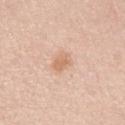<record>
  <site>chest</site>
  <lighting>white-light</lighting>
  <patient>
    <sex>female</sex>
    <age_approx>45</age_approx>
  </patient>
  <lesion_size>
    <long_diameter_mm_approx>2.5</long_diameter_mm_approx>
  </lesion_size>
  <image>
    <source>total-body photography crop</source>
    <field_of_view_mm>15</field_of_view_mm>
  </image>
</record>